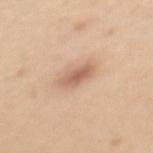biopsy status: no biopsy performed (imaged during a skin exam)
lesion size: ≈3.5 mm
image: ~15 mm tile from a whole-body skin photo
illumination: white-light
patient: female, aged around 30
site: the mid back